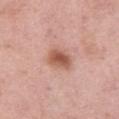Q: Was a biopsy performed?
A: imaged on a skin check; not biopsied
Q: Where on the body is the lesion?
A: the leg
Q: What is the imaging modality?
A: 15 mm crop, total-body photography
Q: What did automated image analysis measure?
A: a mean CIELAB color near L≈56 a*≈25 b*≈29, about 13 CIELAB-L* units darker than the surrounding skin, and a normalized border contrast of about 9
Q: What are the patient's age and sex?
A: female, about 50 years old
Q: Illumination type?
A: white-light illumination
Q: What is the lesion's diameter?
A: ≈3 mm